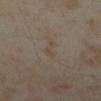Q: Was this lesion biopsied?
A: no biopsy performed (imaged during a skin exam)
Q: Lesion location?
A: the left lower leg
Q: What did automated image analysis measure?
A: about 4 CIELAB-L* units darker than the surrounding skin and a lesion-to-skin contrast of about 5 (normalized; higher = more distinct); a classifier nevus-likeness of about 0/100 and lesion-presence confidence of about 95/100
Q: What are the patient's age and sex?
A: female, aged around 35
Q: What is the imaging modality?
A: total-body-photography crop, ~15 mm field of view